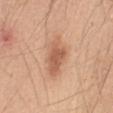<lesion>
  <biopsy_status>not biopsied; imaged during a skin examination</biopsy_status>
  <image>
    <source>total-body photography crop</source>
    <field_of_view_mm>15</field_of_view_mm>
  </image>
  <lighting>white-light</lighting>
  <patient>
    <sex>male</sex>
    <age_approx>50</age_approx>
  </patient>
  <lesion_size>
    <long_diameter_mm_approx>5.5</long_diameter_mm_approx>
  </lesion_size>
  <site>abdomen</site>
</lesion>